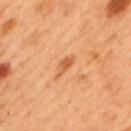size: about 3 mm
image: ~15 mm crop, total-body skin-cancer survey
patient: male, aged approximately 50
anatomic site: the mid back
illumination: cross-polarized
image-analysis metrics: a lesion area of about 3 mm², an outline eccentricity of about 0.9 (0 = round, 1 = elongated), and a shape-asymmetry score of about 0.4 (0 = symmetric); a classifier nevus-likeness of about 5/100